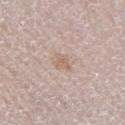Imaged during a routine full-body skin examination; the lesion was not biopsied and no histopathology is available. Located on the right lower leg. A male patient, roughly 60 years of age. Longest diameter approximately 2.5 mm. A roughly 15 mm field-of-view crop from a total-body skin photograph.